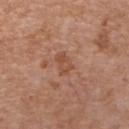Findings:
- workup: no biopsy performed (imaged during a skin exam)
- image source: ~15 mm crop, total-body skin-cancer survey
- anatomic site: the front of the torso
- subject: male, aged around 60